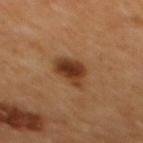Imaged during a routine full-body skin examination; the lesion was not biopsied and no histopathology is available.
This image is a 15 mm lesion crop taken from a total-body photograph.
A male subject.
The total-body-photography lesion software estimated a shape-asymmetry score of about 0.35 (0 = symmetric). The software also gave border irregularity of about 3.5 on a 0–10 scale, internal color variation of about 4.5 on a 0–10 scale, and peripheral color asymmetry of about 1.5.
This is a cross-polarized tile.
Approximately 4.5 mm at its widest.
Located on the mid back.